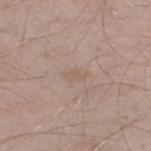follow-up: total-body-photography surveillance lesion; no biopsy
diameter: about 2.5 mm
subject: male, approximately 45 years of age
site: the right thigh
illumination: white-light illumination
image source: 15 mm crop, total-body photography